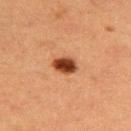Imaged during a routine full-body skin examination; the lesion was not biopsied and no histopathology is available.
The subject is a female aged approximately 40.
This is a cross-polarized tile.
On the upper back.
The lesion's longest dimension is about 3 mm.
A 15 mm crop from a total-body photograph taken for skin-cancer surveillance.